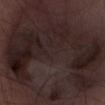The lesion was photographed on a routine skin check and not biopsied; there is no pathology result. The recorded lesion diameter is about 19.5 mm. Imaged with white-light lighting. The patient is a male aged 28 to 32. Cropped from a whole-body photographic skin survey; the tile spans about 15 mm. The lesion is located on the abdomen.